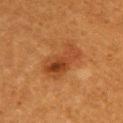Q: Was this lesion biopsied?
A: imaged on a skin check; not biopsied
Q: What is the lesion's diameter?
A: ≈5 mm
Q: What is the imaging modality?
A: ~15 mm crop, total-body skin-cancer survey
Q: What are the patient's age and sex?
A: female, in their mid-50s
Q: Illumination type?
A: cross-polarized illumination
Q: What is the anatomic site?
A: the back